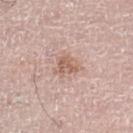workup = no biopsy performed (imaged during a skin exam) | lighting = white-light illumination | imaging modality = ~15 mm crop, total-body skin-cancer survey | lesion size = ~3.5 mm (longest diameter) | patient = male, aged approximately 70 | anatomic site = the right leg.